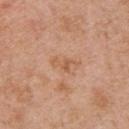<record>
<site>chest</site>
<patient>
  <sex>male</sex>
  <age_approx>70</age_approx>
</patient>
<image>
  <source>total-body photography crop</source>
  <field_of_view_mm>15</field_of_view_mm>
</image>
</record>